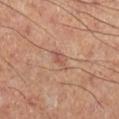Captured during whole-body skin photography for melanoma surveillance; the lesion was not biopsied. Imaged with cross-polarized lighting. A close-up tile cropped from a whole-body skin photograph, about 15 mm across. The lesion's longest dimension is about 3 mm. On the right lower leg. The patient is a male aged 48 to 52.